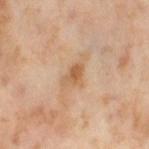No biopsy was performed on this lesion — it was imaged during a full skin examination and was not determined to be concerning.
The recorded lesion diameter is about 2.5 mm.
Located on the right thigh.
A 15 mm crop from a total-body photograph taken for skin-cancer surveillance.
The tile uses cross-polarized illumination.
A female subject in their mid-50s.